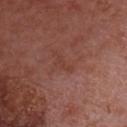Captured during whole-body skin photography for melanoma surveillance; the lesion was not biopsied. The lesion is located on the chest. Cropped from a whole-body photographic skin survey; the tile spans about 15 mm. The subject is a male roughly 65 years of age.